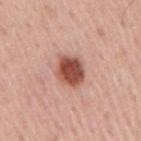Clinical impression: The lesion was tiled from a total-body skin photograph and was not biopsied. Background: The lesion is on the right upper arm. A region of skin cropped from a whole-body photographic capture, roughly 15 mm wide. The subject is a male in their mid- to late 60s. The recorded lesion diameter is about 4 mm.